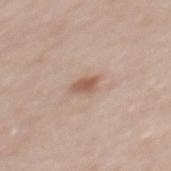{
  "biopsy_status": "not biopsied; imaged during a skin examination",
  "site": "mid back",
  "image": {
    "source": "total-body photography crop",
    "field_of_view_mm": 15
  },
  "patient": {
    "sex": "female",
    "age_approx": 30
  }
}